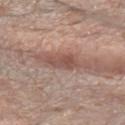This lesion was catalogued during total-body skin photography and was not selected for biopsy.
A female patient, in their mid-60s.
Imaged with white-light lighting.
Cropped from a total-body skin-imaging series; the visible field is about 15 mm.
On the left forearm.
About 3.5 mm across.